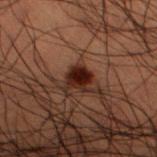Imaged during a routine full-body skin examination; the lesion was not biopsied and no histopathology is available. A male patient, aged 48 to 52. Captured under cross-polarized illumination. This image is a 15 mm lesion crop taken from a total-body photograph. Located on the left lower leg. Automated image analysis of the tile measured a border-irregularity index near 2.5/10, internal color variation of about 3.5 on a 0–10 scale, and a peripheral color-asymmetry measure near 1. Longest diameter approximately 2.5 mm.